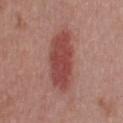The subject is a male aged around 30. About 7.5 mm across. Imaged with white-light lighting. A roughly 15 mm field-of-view crop from a total-body skin photograph.